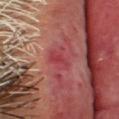diameter = ≈3 mm
image = ~15 mm tile from a whole-body skin photo
image-analysis metrics = a mean CIELAB color near L≈41 a*≈34 b*≈22 and roughly 7 lightness units darker than nearby skin; a border-irregularity rating of about 3.5/10; a classifier nevus-likeness of about 0/100 and a lesion-detection confidence of about 95/100
site = the head or neck
subject = male, aged 68 to 72